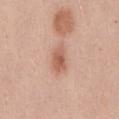The lesion was photographed on a routine skin check and not biopsied; there is no pathology result.